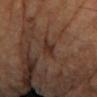The lesion was tiled from a total-body skin photograph and was not biopsied. Imaged with cross-polarized lighting. The subject is a female aged around 70. The lesion is located on the left forearm. Automated image analysis of the tile measured a lesion area of about 3 mm², an outline eccentricity of about 0.8 (0 = round, 1 = elongated), and a symmetry-axis asymmetry near 0.2. It also reported a border-irregularity rating of about 3/10, internal color variation of about 2 on a 0–10 scale, and peripheral color asymmetry of about 0.5. It also reported a nevus-likeness score of about 5/100 and a detector confidence of about 100 out of 100 that the crop contains a lesion. A roughly 15 mm field-of-view crop from a total-body skin photograph.